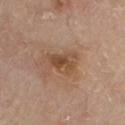Impression: No biopsy was performed on this lesion — it was imaged during a full skin examination and was not determined to be concerning. Background: The lesion's longest dimension is about 3.5 mm. The lesion is located on the front of the torso. Captured under white-light illumination. A male patient, aged around 80. The lesion-visualizer software estimated a border-irregularity index near 3.5/10. The software also gave a classifier nevus-likeness of about 5/100 and a lesion-detection confidence of about 100/100. A region of skin cropped from a whole-body photographic capture, roughly 15 mm wide.